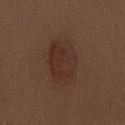Impression:
No biopsy was performed on this lesion — it was imaged during a full skin examination and was not determined to be concerning.
Context:
On the mid back. The lesion-visualizer software estimated an area of roughly 18 mm², an outline eccentricity of about 0.75 (0 = round, 1 = elongated), and a symmetry-axis asymmetry near 0.15. And it measured a lesion–skin lightness drop of about 6 and a normalized lesion–skin contrast near 6. Cropped from a total-body skin-imaging series; the visible field is about 15 mm. Longest diameter approximately 6 mm. A female subject roughly 30 years of age.